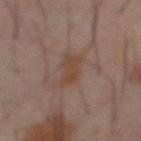{"lesion_size": {"long_diameter_mm_approx": 4.0}, "image": {"source": "total-body photography crop", "field_of_view_mm": 15}, "patient": {"sex": "male", "age_approx": 60}, "lighting": "cross-polarized", "site": "abdomen"}